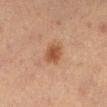notes = total-body-photography surveillance lesion; no biopsy | subject = female, approximately 55 years of age | image-analysis metrics = an average lesion color of about L≈40 a*≈20 b*≈28 (CIELAB), roughly 9 lightness units darker than nearby skin, and a normalized lesion–skin contrast near 8; a border-irregularity rating of about 1/10, internal color variation of about 2.5 on a 0–10 scale, and a peripheral color-asymmetry measure near 1 | size = ≈2.5 mm | tile lighting = cross-polarized illumination | anatomic site = the right lower leg | image source = ~15 mm tile from a whole-body skin photo.